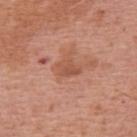biopsy status = total-body-photography surveillance lesion; no biopsy
anatomic site = the upper back
subject = male, in their 70s
diameter = about 2.5 mm
lighting = white-light illumination
TBP lesion metrics = an average lesion color of about L≈53 a*≈25 b*≈32 (CIELAB), roughly 8 lightness units darker than nearby skin, and a normalized border contrast of about 6
image source = 15 mm crop, total-body photography